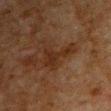Impression:
Captured during whole-body skin photography for melanoma surveillance; the lesion was not biopsied.
Context:
Automated image analysis of the tile measured a lesion color around L≈22 a*≈16 b*≈24 in CIELAB. The software also gave a color-variation rating of about 1.5/10 and radial color variation of about 0.5. The software also gave a classifier nevus-likeness of about 0/100 and lesion-presence confidence of about 100/100. A male subject, in their 80s. The tile uses cross-polarized illumination. On the left upper arm. This image is a 15 mm lesion crop taken from a total-body photograph.